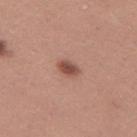Q: Is there a histopathology result?
A: no biopsy performed (imaged during a skin exam)
Q: Who is the patient?
A: female, about 30 years old
Q: What did automated image analysis measure?
A: a footprint of about 4 mm² and two-axis asymmetry of about 0.2; a lesion–skin lightness drop of about 12 and a lesion-to-skin contrast of about 8.5 (normalized; higher = more distinct); border irregularity of about 2 on a 0–10 scale and peripheral color asymmetry of about 1; an automated nevus-likeness rating near 95 out of 100 and a detector confidence of about 100 out of 100 that the crop contains a lesion
Q: How was the tile lit?
A: white-light
Q: Lesion location?
A: the leg
Q: How was this image acquired?
A: 15 mm crop, total-body photography